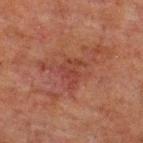The lesion was tiled from a total-body skin photograph and was not biopsied.
A 15 mm close-up extracted from a 3D total-body photography capture.
The lesion's longest dimension is about 3.5 mm.
Automated image analysis of the tile measured border irregularity of about 5.5 on a 0–10 scale and peripheral color asymmetry of about 1.
A male subject, aged 58 to 62.
This is a cross-polarized tile.
The lesion is on the upper back.